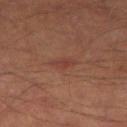Assessment: Imaged during a routine full-body skin examination; the lesion was not biopsied and no histopathology is available. Clinical summary: The patient is a male roughly 65 years of age. The lesion is on the left thigh. About 3.5 mm across. This is a cross-polarized tile. A 15 mm crop from a total-body photograph taken for skin-cancer surveillance.